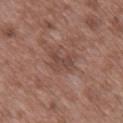No biopsy was performed on this lesion — it was imaged during a full skin examination and was not determined to be concerning. Measured at roughly 3 mm in maximum diameter. An algorithmic analysis of the crop reported a lesion area of about 6 mm², an outline eccentricity of about 0.7 (0 = round, 1 = elongated), and two-axis asymmetry of about 0.45. And it measured a border-irregularity rating of about 5.5/10, a within-lesion color-variation index near 2/10, and a peripheral color-asymmetry measure near 0.5. And it measured lesion-presence confidence of about 100/100. A male patient in their 50s. Located on the back. This is a white-light tile. A 15 mm close-up extracted from a 3D total-body photography capture.